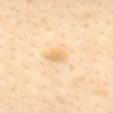Q: How large is the lesion?
A: about 3 mm
Q: What kind of image is this?
A: ~15 mm tile from a whole-body skin photo
Q: Automated lesion metrics?
A: an area of roughly 4 mm² and an eccentricity of roughly 0.8; a border-irregularity rating of about 1.5/10 and internal color variation of about 1.5 on a 0–10 scale; an automated nevus-likeness rating near 0 out of 100 and lesion-presence confidence of about 100/100
Q: Lesion location?
A: the mid back
Q: Who is the patient?
A: female, in their 40s
Q: Illumination type?
A: cross-polarized illumination